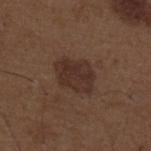{"site": "upper back", "patient": {"sex": "male", "age_approx": 50}, "image": {"source": "total-body photography crop", "field_of_view_mm": 15}}